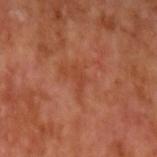Q: Was a biopsy performed?
A: catalogued during a skin exam; not biopsied
Q: What is the imaging modality?
A: ~15 mm crop, total-body skin-cancer survey
Q: What is the anatomic site?
A: the arm
Q: Who is the patient?
A: male, approximately 55 years of age
Q: What is the lesion's diameter?
A: ~2.5 mm (longest diameter)
Q: Illumination type?
A: cross-polarized